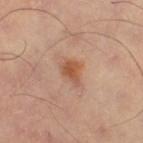automated metrics — a lesion color around L≈54 a*≈24 b*≈34 in CIELAB, a lesion–skin lightness drop of about 10, and a normalized border contrast of about 8 | lesion diameter — ≈3 mm | acquisition — 15 mm crop, total-body photography | illumination — cross-polarized illumination | body site — the leg.